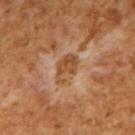automated metrics — a lesion color around L≈48 a*≈21 b*≈35 in CIELAB and about 9 CIELAB-L* units darker than the surrounding skin; a border-irregularity index near 5/10, internal color variation of about 2.5 on a 0–10 scale, and radial color variation of about 1
patient — male, approximately 65 years of age
lighting — cross-polarized
acquisition — ~15 mm tile from a whole-body skin photo